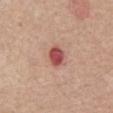Clinical impression: Part of a total-body skin-imaging series; this lesion was reviewed on a skin check and was not flagged for biopsy. Background: On the chest. About 3.5 mm across. An algorithmic analysis of the crop reported an average lesion color of about L≈52 a*≈29 b*≈26 (CIELAB), roughly 14 lightness units darker than nearby skin, and a lesion-to-skin contrast of about 9.5 (normalized; higher = more distinct). It also reported a border-irregularity index near 1.5/10, internal color variation of about 3 on a 0–10 scale, and radial color variation of about 1. The patient is a male aged approximately 65. This is a white-light tile. A close-up tile cropped from a whole-body skin photograph, about 15 mm across.